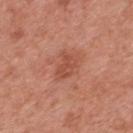Q: Was a biopsy performed?
A: total-body-photography surveillance lesion; no biopsy
Q: Lesion size?
A: ≈3 mm
Q: Where on the body is the lesion?
A: the back
Q: Who is the patient?
A: male, approximately 70 years of age
Q: Automated lesion metrics?
A: a footprint of about 4.5 mm², an outline eccentricity of about 0.75 (0 = round, 1 = elongated), and two-axis asymmetry of about 0.3
Q: What kind of image is this?
A: ~15 mm crop, total-body skin-cancer survey